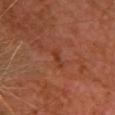Impression: Captured during whole-body skin photography for melanoma surveillance; the lesion was not biopsied. Image and clinical context: About 2.5 mm across. A female subject aged approximately 55. This image is a 15 mm lesion crop taken from a total-body photograph. From the chest. Captured under cross-polarized illumination. An algorithmic analysis of the crop reported a border-irregularity rating of about 5/10, internal color variation of about 0 on a 0–10 scale, and peripheral color asymmetry of about 0.